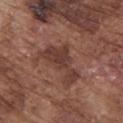Q: Is there a histopathology result?
A: total-body-photography surveillance lesion; no biopsy
Q: What are the patient's age and sex?
A: male, roughly 75 years of age
Q: Lesion location?
A: the chest
Q: What kind of image is this?
A: 15 mm crop, total-body photography
Q: How was the tile lit?
A: white-light illumination
Q: What is the lesion's diameter?
A: ≈5.5 mm